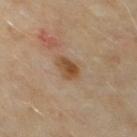The lesion was photographed on a routine skin check and not biopsied; there is no pathology result.
The lesion is located on the mid back.
A close-up tile cropped from a whole-body skin photograph, about 15 mm across.
Automated image analysis of the tile measured a mean CIELAB color near L≈46 a*≈17 b*≈32, about 10 CIELAB-L* units darker than the surrounding skin, and a lesion-to-skin contrast of about 9 (normalized; higher = more distinct). It also reported border irregularity of about 2 on a 0–10 scale and a within-lesion color-variation index near 4/10.
The subject is a male aged 58 to 62.
Measured at roughly 3 mm in maximum diameter.
The tile uses cross-polarized illumination.